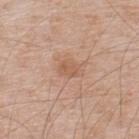Notes:
• workup: no biopsy performed (imaged during a skin exam)
• location: the back
• automated metrics: an area of roughly 5 mm² and an eccentricity of roughly 0.7; an automated nevus-likeness rating near 0 out of 100
• image: total-body-photography crop, ~15 mm field of view
• patient: male, approximately 50 years of age
• lighting: white-light illumination